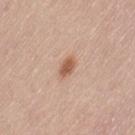Impression: Part of a total-body skin-imaging series; this lesion was reviewed on a skin check and was not flagged for biopsy. Context: Cropped from a whole-body photographic skin survey; the tile spans about 15 mm. Imaged with white-light lighting. Automated image analysis of the tile measured a footprint of about 3.5 mm² and an outline eccentricity of about 0.8 (0 = round, 1 = elongated). The analysis additionally found a border-irregularity index near 2.5/10, a color-variation rating of about 2.5/10, and a peripheral color-asymmetry measure near 1. The analysis additionally found an automated nevus-likeness rating near 95 out of 100 and a detector confidence of about 100 out of 100 that the crop contains a lesion. Located on the left thigh. A female patient, roughly 65 years of age.